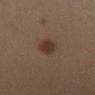follow-up = catalogued during a skin exam; not biopsied | image source = ~15 mm crop, total-body skin-cancer survey | subject = female, approximately 35 years of age | site = the right thigh.